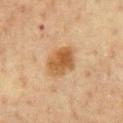No biopsy was performed on this lesion — it was imaged during a full skin examination and was not determined to be concerning. On the front of the torso. The subject is a male aged 58 to 62. This is a cross-polarized tile. An algorithmic analysis of the crop reported an average lesion color of about L≈50 a*≈18 b*≈36 (CIELAB), about 11 CIELAB-L* units darker than the surrounding skin, and a normalized border contrast of about 8.5. About 4.5 mm across. Cropped from a total-body skin-imaging series; the visible field is about 15 mm.